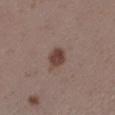The lesion was tiled from a total-body skin photograph and was not biopsied.
From the left lower leg.
A female patient, in their 30s.
A lesion tile, about 15 mm wide, cut from a 3D total-body photograph.